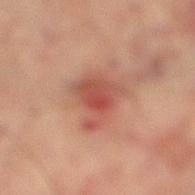Q: Is there a histopathology result?
A: imaged on a skin check; not biopsied
Q: Where on the body is the lesion?
A: the right lower leg
Q: How large is the lesion?
A: ~4.5 mm (longest diameter)
Q: How was this image acquired?
A: total-body-photography crop, ~15 mm field of view
Q: Who is the patient?
A: male, approximately 75 years of age
Q: How was the tile lit?
A: cross-polarized illumination